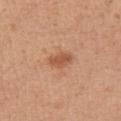Impression:
No biopsy was performed on this lesion — it was imaged during a full skin examination and was not determined to be concerning.
Background:
A roughly 15 mm field-of-view crop from a total-body skin photograph. From the left upper arm. The patient is a female aged 43–47. The total-body-photography lesion software estimated an area of roughly 5 mm², an eccentricity of roughly 0.75, and a shape-asymmetry score of about 0.25 (0 = symmetric). The analysis additionally found an average lesion color of about L≈55 a*≈24 b*≈34 (CIELAB), a lesion–skin lightness drop of about 10, and a normalized lesion–skin contrast near 7. And it measured a nevus-likeness score of about 80/100.